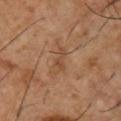This lesion was catalogued during total-body skin photography and was not selected for biopsy.
From the chest.
A region of skin cropped from a whole-body photographic capture, roughly 15 mm wide.
A male patient, aged 53–57.